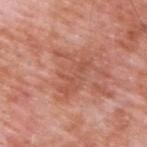{
  "biopsy_status": "not biopsied; imaged during a skin examination",
  "image": {
    "source": "total-body photography crop",
    "field_of_view_mm": 15
  },
  "lighting": "white-light",
  "patient": {
    "sex": "male",
    "age_approx": 60
  },
  "site": "upper back",
  "lesion_size": {
    "long_diameter_mm_approx": 5.0
  }
}